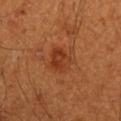Assessment:
The lesion was photographed on a routine skin check and not biopsied; there is no pathology result.
Acquisition and patient details:
The recorded lesion diameter is about 3 mm. This is a cross-polarized tile. A male patient, aged 58 to 62. The lesion is located on the left upper arm. A 15 mm close-up tile from a total-body photography series done for melanoma screening.